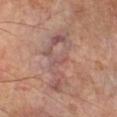workup: no biopsy performed (imaged during a skin exam)
subject: male, aged 68–72
imaging modality: 15 mm crop, total-body photography
location: the right lower leg